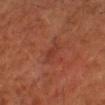size = ~2.5 mm (longest diameter) | subject = male, roughly 60 years of age | tile lighting = cross-polarized | anatomic site = the right upper arm | acquisition = ~15 mm crop, total-body skin-cancer survey.